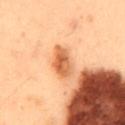Captured during whole-body skin photography for melanoma surveillance; the lesion was not biopsied.